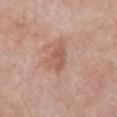Captured during whole-body skin photography for melanoma surveillance; the lesion was not biopsied. A male patient, approximately 80 years of age. The lesion is located on the chest. The tile uses white-light illumination. A 15 mm close-up tile from a total-body photography series done for melanoma screening.